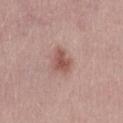Clinical impression:
Captured during whole-body skin photography for melanoma surveillance; the lesion was not biopsied.
Acquisition and patient details:
Cropped from a whole-body photographic skin survey; the tile spans about 15 mm. A male patient in their 30s. Measured at roughly 3 mm in maximum diameter. The lesion-visualizer software estimated an area of roughly 5.5 mm², a shape eccentricity near 0.6, and a symmetry-axis asymmetry near 0.3. The analysis additionally found an automated nevus-likeness rating near 70 out of 100.